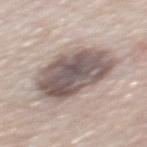No biopsy was performed on this lesion — it was imaged during a full skin examination and was not determined to be concerning.
Automated tile analysis of the lesion measured a footprint of about 31 mm², an eccentricity of roughly 0.7, and two-axis asymmetry of about 0.15. And it measured an average lesion color of about L≈54 a*≈12 b*≈18 (CIELAB), about 15 CIELAB-L* units darker than the surrounding skin, and a normalized lesion–skin contrast near 10.5. And it measured a color-variation rating of about 6.5/10 and a peripheral color-asymmetry measure near 2.5. It also reported a nevus-likeness score of about 20/100 and lesion-presence confidence of about 20/100.
From the mid back.
A male subject, roughly 65 years of age.
A roughly 15 mm field-of-view crop from a total-body skin photograph.
About 7.5 mm across.